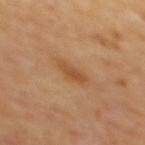Imaged during a routine full-body skin examination; the lesion was not biopsied and no histopathology is available. An algorithmic analysis of the crop reported a lesion area of about 5 mm², an outline eccentricity of about 0.8 (0 = round, 1 = elongated), and a symmetry-axis asymmetry near 0.3. And it measured an average lesion color of about L≈50 a*≈21 b*≈37 (CIELAB), a lesion–skin lightness drop of about 8, and a normalized lesion–skin contrast near 6.5. The analysis additionally found a border-irregularity rating of about 3/10, a color-variation rating of about 2/10, and peripheral color asymmetry of about 0.5. A male subject aged around 65. The tile uses cross-polarized illumination. The lesion is located on the mid back. A roughly 15 mm field-of-view crop from a total-body skin photograph.